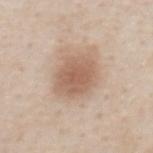Impression:
Recorded during total-body skin imaging; not selected for excision or biopsy.
Clinical summary:
Cropped from a whole-body photographic skin survey; the tile spans about 15 mm. A male patient, approximately 60 years of age. The lesion is located on the chest.